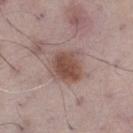biopsy status: imaged on a skin check; not biopsied
acquisition: ~15 mm crop, total-body skin-cancer survey
subject: male, aged 68–72
size: ~3.5 mm (longest diameter)
location: the right thigh